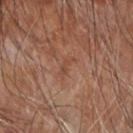Assessment: The lesion was photographed on a routine skin check and not biopsied; there is no pathology result. Acquisition and patient details: An algorithmic analysis of the crop reported a footprint of about 2 mm² and a shape eccentricity near 0.9. And it measured a lesion color around L≈44 a*≈22 b*≈29 in CIELAB, roughly 6 lightness units darker than nearby skin, and a normalized border contrast of about 5. The recorded lesion diameter is about 2.5 mm. A 15 mm close-up extracted from a 3D total-body photography capture. A male subject aged approximately 70. On the right forearm.